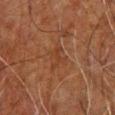Part of a total-body skin-imaging series; this lesion was reviewed on a skin check and was not flagged for biopsy.
The lesion is located on the chest.
The subject is a male in their 60s.
A lesion tile, about 15 mm wide, cut from a 3D total-body photograph.